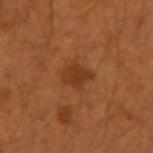follow-up: catalogued during a skin exam; not biopsied
site: the left upper arm
image: ~15 mm crop, total-body skin-cancer survey
patient: male, roughly 50 years of age